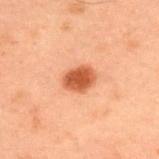biopsy_status: not biopsied; imaged during a skin examination
patient:
  sex: male
  age_approx: 55
site: upper back
lighting: cross-polarized
automated_metrics:
  border_irregularity_0_10: 1.0
  color_variation_0_10: 2.5
  nevus_likeness_0_100: 100
  lesion_detection_confidence_0_100: 100
image:
  source: total-body photography crop
  field_of_view_mm: 15
lesion_size:
  long_diameter_mm_approx: 3.0